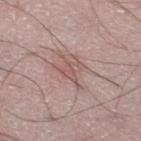Q: Is there a histopathology result?
A: total-body-photography surveillance lesion; no biopsy
Q: How large is the lesion?
A: ≈4 mm
Q: What are the patient's age and sex?
A: male, about 50 years old
Q: What is the imaging modality?
A: 15 mm crop, total-body photography
Q: What is the anatomic site?
A: the leg